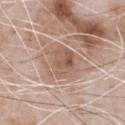No biopsy was performed on this lesion — it was imaged during a full skin examination and was not determined to be concerning.
Cropped from a whole-body photographic skin survey; the tile spans about 15 mm.
The lesion-visualizer software estimated an area of roughly 19 mm², an outline eccentricity of about 0.7 (0 = round, 1 = elongated), and a shape-asymmetry score of about 0.3 (0 = symmetric). The software also gave a lesion color around L≈59 a*≈17 b*≈27 in CIELAB, a lesion–skin lightness drop of about 10, and a normalized border contrast of about 7. And it measured a border-irregularity index near 3.5/10, a within-lesion color-variation index near 5.5/10, and peripheral color asymmetry of about 1.5.
A male patient roughly 50 years of age.
Approximately 6 mm at its widest.
The lesion is on the chest.
Captured under white-light illumination.